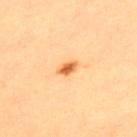Notes:
- follow-up · imaged on a skin check; not biopsied
- site · the upper back
- subject · male, approximately 65 years of age
- TBP lesion metrics · roughly 14 lightness units darker than nearby skin and a lesion-to-skin contrast of about 9 (normalized; higher = more distinct); internal color variation of about 4 on a 0–10 scale; lesion-presence confidence of about 100/100
- image source · ~15 mm crop, total-body skin-cancer survey
- lighting · cross-polarized illumination
- diameter · about 3 mm